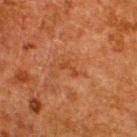This lesion was catalogued during total-body skin photography and was not selected for biopsy. The lesion is on the upper back. Longest diameter approximately 2.5 mm. Cropped from a whole-body photographic skin survey; the tile spans about 15 mm. A male patient aged around 60.